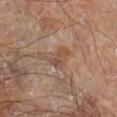Imaged during a routine full-body skin examination; the lesion was not biopsied and no histopathology is available. The lesion is located on the right lower leg. Imaged with cross-polarized lighting. Cropped from a whole-body photographic skin survey; the tile spans about 15 mm. Measured at roughly 3 mm in maximum diameter. A male subject roughly 70 years of age.